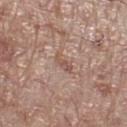Q: Is there a histopathology result?
A: catalogued during a skin exam; not biopsied
Q: What is the anatomic site?
A: the right lower leg
Q: What did automated image analysis measure?
A: a lesion color around L≈53 a*≈19 b*≈25 in CIELAB and roughly 8 lightness units darker than nearby skin; border irregularity of about 3.5 on a 0–10 scale and a color-variation rating of about 1/10
Q: How was this image acquired?
A: ~15 mm crop, total-body skin-cancer survey
Q: Who is the patient?
A: male, aged around 70
Q: What is the lesion's diameter?
A: about 2.5 mm